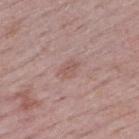Recorded during total-body skin imaging; not selected for excision or biopsy. The lesion is located on the upper back. Longest diameter approximately 2.5 mm. A female patient, approximately 50 years of age. A lesion tile, about 15 mm wide, cut from a 3D total-body photograph.